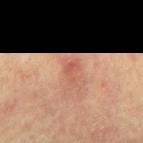Assessment: This lesion was catalogued during total-body skin photography and was not selected for biopsy. Context: Cropped from a total-body skin-imaging series; the visible field is about 15 mm. A male patient in their mid-60s. Automated image analysis of the tile measured a border-irregularity index near 3.5/10 and radial color variation of about 0.5. The software also gave a nevus-likeness score of about 0/100. About 2.5 mm across. The tile uses cross-polarized illumination. On the mid back.